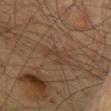Assessment: No biopsy was performed on this lesion — it was imaged during a full skin examination and was not determined to be concerning. Background: Captured under cross-polarized illumination. Located on the back. Cropped from a whole-body photographic skin survey; the tile spans about 15 mm. The recorded lesion diameter is about 4 mm. A male subject, aged 58 to 62.